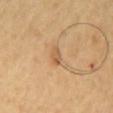A close-up tile cropped from a whole-body skin photograph, about 15 mm across.
Automated tile analysis of the lesion measured an outline eccentricity of about 0.85 (0 = round, 1 = elongated) and two-axis asymmetry of about 0.1. And it measured border irregularity of about 1 on a 0–10 scale and internal color variation of about 1.5 on a 0–10 scale. And it measured a classifier nevus-likeness of about 0/100 and a lesion-detection confidence of about 100/100.
On the mid back.
The subject is a male aged around 65.
Captured under cross-polarized illumination.
Approximately 2.5 mm at its widest.